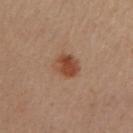Cropped from a total-body skin-imaging series; the visible field is about 15 mm.
The patient is a female aged around 30.
Imaged with cross-polarized lighting.
On the arm.